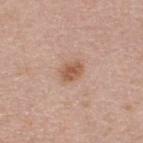No biopsy was performed on this lesion — it was imaged during a full skin examination and was not determined to be concerning. The recorded lesion diameter is about 2.5 mm. A male patient, approximately 45 years of age. The lesion is on the upper back. This image is a 15 mm lesion crop taken from a total-body photograph.